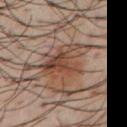Assessment:
Part of a total-body skin-imaging series; this lesion was reviewed on a skin check and was not flagged for biopsy.
Image and clinical context:
Imaged with cross-polarized lighting. Located on the chest. An algorithmic analysis of the crop reported roughly 9 lightness units darker than nearby skin and a normalized lesion–skin contrast near 7.5. It also reported a border-irregularity rating of about 5.5/10, a color-variation rating of about 8/10, and peripheral color asymmetry of about 3. The patient is a male about 40 years old. This image is a 15 mm lesion crop taken from a total-body photograph. About 5.5 mm across.